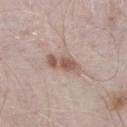The lesion was photographed on a routine skin check and not biopsied; there is no pathology result. Imaged with white-light lighting. The total-body-photography lesion software estimated a border-irregularity index near 3/10 and peripheral color asymmetry of about 1.5. The analysis additionally found a nevus-likeness score of about 90/100 and a lesion-detection confidence of about 100/100. A male subject aged approximately 40. A close-up tile cropped from a whole-body skin photograph, about 15 mm across. On the right lower leg. The lesion's longest dimension is about 4 mm.